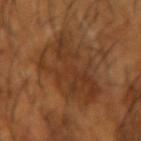Assessment:
Imaged during a routine full-body skin examination; the lesion was not biopsied and no histopathology is available.
Clinical summary:
A 15 mm crop from a total-body photograph taken for skin-cancer surveillance. Measured at roughly 8 mm in maximum diameter. Imaged with cross-polarized lighting. Automated image analysis of the tile measured a lesion area of about 32 mm² and two-axis asymmetry of about 0.35. The analysis additionally found about 7 CIELAB-L* units darker than the surrounding skin and a lesion-to-skin contrast of about 6.5 (normalized; higher = more distinct). The analysis additionally found a classifier nevus-likeness of about 0/100 and a lesion-detection confidence of about 70/100. A male patient, aged 63 to 67. On the left forearm.